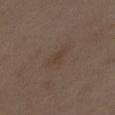The lesion-visualizer software estimated an outline eccentricity of about 0.9 (0 = round, 1 = elongated) and a shape-asymmetry score of about 0.3 (0 = symmetric). And it measured internal color variation of about 1 on a 0–10 scale and peripheral color asymmetry of about 0.5. A 15 mm close-up tile from a total-body photography series done for melanoma screening. The recorded lesion diameter is about 3.5 mm. The lesion is on the abdomen. This is a white-light tile. The subject is a female aged approximately 35.